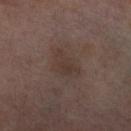automated lesion analysis — border irregularity of about 3.5 on a 0–10 scale, a color-variation rating of about 3/10, and a peripheral color-asymmetry measure near 1; acquisition — total-body-photography crop, ~15 mm field of view; anatomic site — the right thigh; patient — female, in their mid- to late 50s; lighting — cross-polarized.